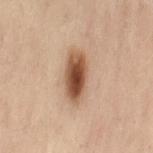Part of a total-body skin-imaging series; this lesion was reviewed on a skin check and was not flagged for biopsy. A female subject roughly 55 years of age. This is a cross-polarized tile. This image is a 15 mm lesion crop taken from a total-body photograph. On the lower back.